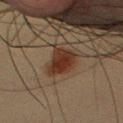Captured during whole-body skin photography for melanoma surveillance; the lesion was not biopsied. A male patient, aged 58 to 62. Imaged with cross-polarized lighting. From the chest. A close-up tile cropped from a whole-body skin photograph, about 15 mm across. The total-body-photography lesion software estimated an automated nevus-likeness rating near 100 out of 100 and a detector confidence of about 100 out of 100 that the crop contains a lesion. Longest diameter approximately 4 mm.